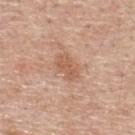Impression:
No biopsy was performed on this lesion — it was imaged during a full skin examination and was not determined to be concerning.
Background:
From the upper back. An algorithmic analysis of the crop reported an area of roughly 6 mm² and a shape-asymmetry score of about 0.25 (0 = symmetric). And it measured an automated nevus-likeness rating near 5 out of 100 and lesion-presence confidence of about 100/100. About 3.5 mm across. This is a white-light tile. A male subject in their mid-50s. A 15 mm close-up extracted from a 3D total-body photography capture.